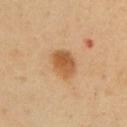Part of a total-body skin-imaging series; this lesion was reviewed on a skin check and was not flagged for biopsy. Cropped from a total-body skin-imaging series; the visible field is about 15 mm. A male subject aged 48–52. Approximately 3.5 mm at its widest. Located on the abdomen.